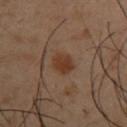Background: A male subject aged 38 to 42. On the upper back. The tile uses cross-polarized illumination. A lesion tile, about 15 mm wide, cut from a 3D total-body photograph.